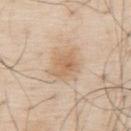Imaged during a routine full-body skin examination; the lesion was not biopsied and no histopathology is available.